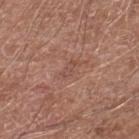image-analysis metrics: a lesion area of about 2.5 mm², an outline eccentricity of about 0.9 (0 = round, 1 = elongated), and a shape-asymmetry score of about 0.35 (0 = symmetric); a mean CIELAB color near L≈49 a*≈21 b*≈25, a lesion–skin lightness drop of about 6, and a normalized lesion–skin contrast near 4.5; a border-irregularity rating of about 3.5/10 and radial color variation of about 0 | image: total-body-photography crop, ~15 mm field of view | patient: male, aged 78–82 | location: the leg.